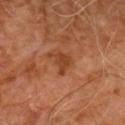No biopsy was performed on this lesion — it was imaged during a full skin examination and was not determined to be concerning.
This is a cross-polarized tile.
Automated image analysis of the tile measured an eccentricity of roughly 0.75 and a symmetry-axis asymmetry near 0.5. It also reported a lesion color around L≈40 a*≈25 b*≈35 in CIELAB and a normalized border contrast of about 7. The analysis additionally found border irregularity of about 4.5 on a 0–10 scale, a within-lesion color-variation index near 1/10, and peripheral color asymmetry of about 0.5. It also reported an automated nevus-likeness rating near 0 out of 100.
The lesion is located on the chest.
The patient is a male in their 60s.
Cropped from a total-body skin-imaging series; the visible field is about 15 mm.